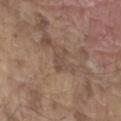From the left forearm. A 15 mm crop from a total-body photograph taken for skin-cancer surveillance. Imaged with white-light lighting. The recorded lesion diameter is about 3 mm. A male subject, aged 78–82. The lesion-visualizer software estimated an area of roughly 4 mm² and an outline eccentricity of about 0.7 (0 = round, 1 = elongated). And it measured border irregularity of about 4 on a 0–10 scale, a within-lesion color-variation index near 1.5/10, and radial color variation of about 0.5.